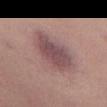imaging modality: total-body-photography crop, ~15 mm field of view
anatomic site: the left thigh
diameter: ≈7 mm
tile lighting: white-light illumination
automated lesion analysis: an area of roughly 20 mm², a shape eccentricity near 0.85, and two-axis asymmetry of about 0.2; a classifier nevus-likeness of about 5/100 and lesion-presence confidence of about 100/100
patient: female, aged around 45